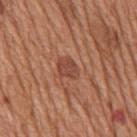follow-up — no biopsy performed (imaged during a skin exam) | patient — male, aged around 65 | location — the mid back | acquisition — ~15 mm crop, total-body skin-cancer survey.